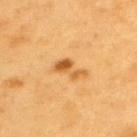Assessment:
The lesion was photographed on a routine skin check and not biopsied; there is no pathology result.
Clinical summary:
A male patient, aged approximately 60. The lesion's longest dimension is about 3.5 mm. This image is a 15 mm lesion crop taken from a total-body photograph. On the upper back.